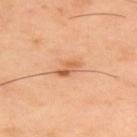{"biopsy_status": "not biopsied; imaged during a skin examination", "image": {"source": "total-body photography crop", "field_of_view_mm": 15}, "automated_metrics": {"area_mm2_approx": 3.0, "eccentricity": 0.9, "shape_asymmetry": 0.35, "border_irregularity_0_10": 3.5, "color_variation_0_10": 1.0, "nevus_likeness_0_100": 50, "lesion_detection_confidence_0_100": 100}, "patient": {"sex": "male", "age_approx": 45}, "lighting": "cross-polarized", "lesion_size": {"long_diameter_mm_approx": 3.0}, "site": "upper back"}